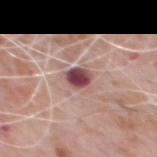notes: no biopsy performed (imaged during a skin exam); lesion size: ≈2.5 mm; body site: the chest; subject: male, about 80 years old; acquisition: ~15 mm tile from a whole-body skin photo.